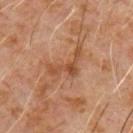follow-up = total-body-photography surveillance lesion; no biopsy
anatomic site = the chest
lighting = cross-polarized illumination
imaging modality = total-body-photography crop, ~15 mm field of view
patient = male, aged approximately 60